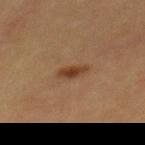notes=total-body-photography surveillance lesion; no biopsy
body site=the mid back
subject=female, about 40 years old
acquisition=total-body-photography crop, ~15 mm field of view